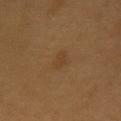biopsy status: catalogued during a skin exam; not biopsied | illumination: cross-polarized | subject: male, about 60 years old | site: the chest | imaging modality: ~15 mm crop, total-body skin-cancer survey | lesion size: ~2.5 mm (longest diameter) | automated lesion analysis: a footprint of about 3.5 mm² and a symmetry-axis asymmetry near 0.2; a border-irregularity index near 2/10, a within-lesion color-variation index near 1/10, and peripheral color asymmetry of about 0.5; lesion-presence confidence of about 100/100.